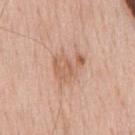The lesion was tiled from a total-body skin photograph and was not biopsied.
About 3.5 mm across.
The tile uses white-light illumination.
From the front of the torso.
A 15 mm crop from a total-body photograph taken for skin-cancer surveillance.
The patient is a male roughly 60 years of age.